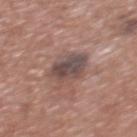Q: Was this lesion biopsied?
A: catalogued during a skin exam; not biopsied
Q: What is the lesion's diameter?
A: ~4 mm (longest diameter)
Q: What is the anatomic site?
A: the chest
Q: What is the imaging modality?
A: 15 mm crop, total-body photography
Q: Patient demographics?
A: male, roughly 45 years of age
Q: Automated lesion metrics?
A: a footprint of about 12 mm², a shape eccentricity near 0.4, and a symmetry-axis asymmetry near 0.15; a border-irregularity rating of about 2/10 and internal color variation of about 6 on a 0–10 scale; an automated nevus-likeness rating near 5 out of 100 and a detector confidence of about 100 out of 100 that the crop contains a lesion
Q: Illumination type?
A: white-light illumination